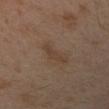{
  "biopsy_status": "not biopsied; imaged during a skin examination",
  "site": "arm",
  "image": {
    "source": "total-body photography crop",
    "field_of_view_mm": 15
  },
  "patient": {
    "sex": "female",
    "age_approx": 40
  }
}